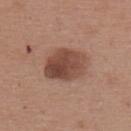Background: From the upper back. A roughly 15 mm field-of-view crop from a total-body skin photograph. The lesion's longest dimension is about 5.5 mm. The lesion-visualizer software estimated an area of roughly 17 mm², an eccentricity of roughly 0.75, and a shape-asymmetry score of about 0.15 (0 = symmetric). And it measured a lesion color around L≈47 a*≈21 b*≈27 in CIELAB, roughly 12 lightness units darker than nearby skin, and a normalized lesion–skin contrast near 9. The analysis additionally found a nevus-likeness score of about 90/100. A female patient roughly 60 years of age. Imaged with white-light lighting.